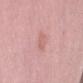{"biopsy_status": "not biopsied; imaged during a skin examination", "image": {"source": "total-body photography crop", "field_of_view_mm": 15}, "lesion_size": {"long_diameter_mm_approx": 3.5}, "patient": {"sex": "female", "age_approx": 40}, "site": "arm", "lighting": "white-light"}